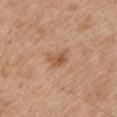<lesion>
  <biopsy_status>not biopsied; imaged during a skin examination</biopsy_status>
  <lesion_size>
    <long_diameter_mm_approx>3.0</long_diameter_mm_approx>
  </lesion_size>
  <lighting>white-light</lighting>
  <patient>
    <sex>male</sex>
    <age_approx>65</age_approx>
  </patient>
  <site>arm</site>
  <automated_metrics>
    <border_irregularity_0_10>4.0</border_irregularity_0_10>
  </automated_metrics>
  <image>
    <source>total-body photography crop</source>
    <field_of_view_mm>15</field_of_view_mm>
  </image>
</lesion>